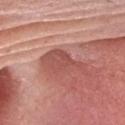{
  "biopsy_status": "not biopsied; imaged during a skin examination",
  "image": {
    "source": "total-body photography crop",
    "field_of_view_mm": 15
  },
  "automated_metrics": {
    "eccentricity": 0.95,
    "shape_asymmetry": 0.45,
    "border_irregularity_0_10": 6.5,
    "color_variation_0_10": 2.0,
    "peripheral_color_asymmetry": 0.5
  },
  "site": "head or neck",
  "lesion_size": {
    "long_diameter_mm_approx": 7.0
  },
  "lighting": "white-light",
  "patient": {
    "sex": "male",
    "age_approx": 60
  }
}